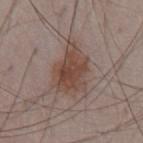  biopsy_status: not biopsied; imaged during a skin examination
  site: abdomen
  patient:
    sex: male
    age_approx: 65
  image:
    source: total-body photography crop
    field_of_view_mm: 15